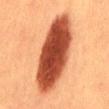notes = imaged on a skin check; not biopsied
image = ~15 mm tile from a whole-body skin photo
illumination = cross-polarized
anatomic site = the back
subject = male, in their 40s
size = ≈11 mm